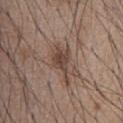Q: Is there a histopathology result?
A: no biopsy performed (imaged during a skin exam)
Q: Patient demographics?
A: male, approximately 55 years of age
Q: What did automated image analysis measure?
A: a footprint of about 8 mm² and a shape eccentricity near 0.9; an average lesion color of about L≈44 a*≈16 b*≈24 (CIELAB), about 10 CIELAB-L* units darker than the surrounding skin, and a normalized border contrast of about 8
Q: How was this image acquired?
A: 15 mm crop, total-body photography
Q: What is the anatomic site?
A: the front of the torso
Q: Illumination type?
A: white-light illumination
Q: What is the lesion's diameter?
A: ≈5.5 mm